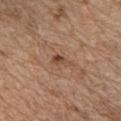• notes · total-body-photography surveillance lesion; no biopsy
• image source · 15 mm crop, total-body photography
• site · the chest
• subject · male, approximately 70 years of age
• lesion size · about 2.5 mm
• automated lesion analysis · an average lesion color of about L≈46 a*≈19 b*≈30 (CIELAB) and a lesion-to-skin contrast of about 7.5 (normalized; higher = more distinct); a border-irregularity rating of about 4/10 and internal color variation of about 2.5 on a 0–10 scale; a lesion-detection confidence of about 100/100
• tile lighting · white-light